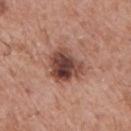This is a white-light tile. The patient is a male roughly 65 years of age. A 15 mm close-up extracted from a 3D total-body photography capture. From the upper back.